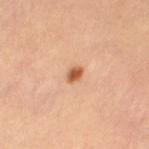This image is a 15 mm lesion crop taken from a total-body photograph. The patient is a female aged 33 to 37. The lesion is on the left thigh.